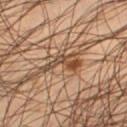Impression: The lesion was tiled from a total-body skin photograph and was not biopsied. Context: A male subject, aged 43–47. Captured under cross-polarized illumination. The recorded lesion diameter is about 5 mm. A 15 mm close-up tile from a total-body photography series done for melanoma screening. Located on the left thigh.